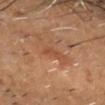notes = catalogued during a skin exam; not biopsied
imaging modality = total-body-photography crop, ~15 mm field of view
patient = male, about 50 years old
lighting = cross-polarized illumination
diameter = ~2.5 mm (longest diameter)
body site = the head or neck